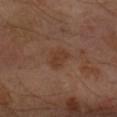• notes — imaged on a skin check; not biopsied
• image — 15 mm crop, total-body photography
• site — the right forearm
• subject — male, approximately 65 years of age
• automated lesion analysis — a footprint of about 6 mm², a shape eccentricity near 0.75, and a symmetry-axis asymmetry near 0.2; border irregularity of about 2 on a 0–10 scale
• lesion diameter — ~3.5 mm (longest diameter)